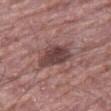Case summary:
* workup: imaged on a skin check; not biopsied
* subject: male, aged 68 to 72
* size: ~4 mm (longest diameter)
* site: the right thigh
* image source: ~15 mm crop, total-body skin-cancer survey